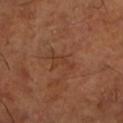Clinical impression: The lesion was photographed on a routine skin check and not biopsied; there is no pathology result. Image and clinical context: Automated tile analysis of the lesion measured an automated nevus-likeness rating near 0 out of 100 and a lesion-detection confidence of about 100/100. Measured at roughly 3.5 mm in maximum diameter. A male subject in their mid- to late 60s. From the left lower leg. A 15 mm close-up extracted from a 3D total-body photography capture. This is a cross-polarized tile.